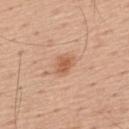Imaged during a routine full-body skin examination; the lesion was not biopsied and no histopathology is available. From the mid back. Captured under white-light illumination. The lesion's longest dimension is about 2.5 mm. A close-up tile cropped from a whole-body skin photograph, about 15 mm across. The patient is a male in their mid- to late 50s.